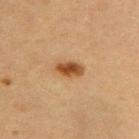The total-body-photography lesion software estimated an area of roughly 5 mm², an outline eccentricity of about 0.85 (0 = round, 1 = elongated), and a symmetry-axis asymmetry near 0.25. And it measured a lesion-detection confidence of about 100/100. Cropped from a total-body skin-imaging series; the visible field is about 15 mm. A male subject, aged 58–62. Located on the back.